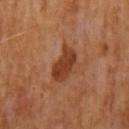follow-up: catalogued during a skin exam; not biopsied | lesion size: about 4.5 mm | image-analysis metrics: an area of roughly 8 mm², an outline eccentricity of about 0.85 (0 = round, 1 = elongated), and two-axis asymmetry of about 0.25; a lesion–skin lightness drop of about 11 and a lesion-to-skin contrast of about 9.5 (normalized; higher = more distinct) | patient: male, aged approximately 65 | illumination: cross-polarized | acquisition: total-body-photography crop, ~15 mm field of view.